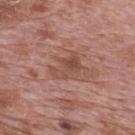biopsy status: imaged on a skin check; not biopsied | tile lighting: white-light | subject: male, aged approximately 70 | imaging modality: ~15 mm tile from a whole-body skin photo | body site: the mid back | TBP lesion metrics: an outline eccentricity of about 0.8 (0 = round, 1 = elongated) and a symmetry-axis asymmetry near 0.45.